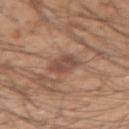Recorded during total-body skin imaging; not selected for excision or biopsy.
From the right upper arm.
The lesion-visualizer software estimated an area of roughly 6 mm², a shape eccentricity near 0.55, and two-axis asymmetry of about 0.2.
Imaged with white-light lighting.
A 15 mm close-up extracted from a 3D total-body photography capture.
A male patient roughly 20 years of age.
Measured at roughly 3 mm in maximum diameter.